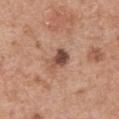notes=total-body-photography surveillance lesion; no biopsy
automated metrics=a lesion area of about 4.5 mm², an eccentricity of roughly 0.7, and a symmetry-axis asymmetry near 0.3; an average lesion color of about L≈48 a*≈20 b*≈27 (CIELAB) and roughly 14 lightness units darker than nearby skin; border irregularity of about 3 on a 0–10 scale and a color-variation rating of about 5/10
diameter=about 3 mm
imaging modality=15 mm crop, total-body photography
body site=the left upper arm
subject=male, approximately 65 years of age